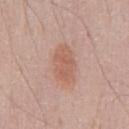Q: Was this lesion biopsied?
A: imaged on a skin check; not biopsied
Q: Lesion size?
A: ~4.5 mm (longest diameter)
Q: What kind of image is this?
A: 15 mm crop, total-body photography
Q: Lesion location?
A: the chest
Q: Who is the patient?
A: male, aged 48 to 52
Q: How was the tile lit?
A: white-light illumination